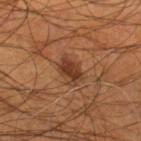Case summary:
– workup — imaged on a skin check; not biopsied
– subject — male, aged approximately 55
– lighting — cross-polarized illumination
– image source — ~15 mm crop, total-body skin-cancer survey
– automated metrics — an average lesion color of about L≈34 a*≈21 b*≈29 (CIELAB), a lesion–skin lightness drop of about 10, and a lesion-to-skin contrast of about 9 (normalized; higher = more distinct)
– location — the right lower leg